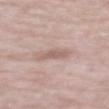Impression:
Recorded during total-body skin imaging; not selected for excision or biopsy.
Acquisition and patient details:
Automated image analysis of the tile measured a footprint of about 4 mm² and a symmetry-axis asymmetry near 0.25. The software also gave an average lesion color of about L≈60 a*≈18 b*≈24 (CIELAB). The analysis additionally found an automated nevus-likeness rating near 5 out of 100 and lesion-presence confidence of about 100/100. A male subject, aged 63 to 67. Longest diameter approximately 3 mm. On the back. A 15 mm close-up tile from a total-body photography series done for melanoma screening. Imaged with white-light lighting.